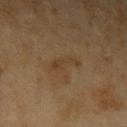Notes:
* workup: catalogued during a skin exam; not biopsied
* automated lesion analysis: a lesion area of about 4 mm² and an eccentricity of roughly 0.95; border irregularity of about 6.5 on a 0–10 scale and a color-variation rating of about 0/10
* image: ~15 mm crop, total-body skin-cancer survey
* subject: female, aged around 60
* anatomic site: the left upper arm
* lighting: cross-polarized
* lesion diameter: ≈4 mm